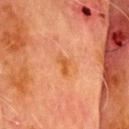This lesion was catalogued during total-body skin photography and was not selected for biopsy.
Cropped from a whole-body photographic skin survey; the tile spans about 15 mm.
The lesion is located on the head or neck.
The recorded lesion diameter is about 2.5 mm.
An algorithmic analysis of the crop reported a lesion area of about 3 mm², an eccentricity of roughly 0.85, and a symmetry-axis asymmetry near 0.35. The analysis additionally found a mean CIELAB color near L≈47 a*≈26 b*≈39, about 7 CIELAB-L* units darker than the surrounding skin, and a normalized lesion–skin contrast near 7.5. And it measured a nevus-likeness score of about 20/100 and a lesion-detection confidence of about 100/100.
The tile uses cross-polarized illumination.
A male patient aged around 70.